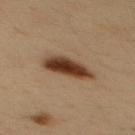biopsy status: no biopsy performed (imaged during a skin exam)
subject: male, in their mid-30s
image source: ~15 mm tile from a whole-body skin photo
lighting: cross-polarized
automated lesion analysis: an area of roughly 11 mm², a shape eccentricity near 0.9, and a shape-asymmetry score of about 0.2 (0 = symmetric); a mean CIELAB color near L≈35 a*≈17 b*≈27, about 16 CIELAB-L* units darker than the surrounding skin, and a lesion-to-skin contrast of about 13.5 (normalized; higher = more distinct)
body site: the mid back